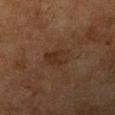Longest diameter approximately 3.5 mm. On the left upper arm. A roughly 15 mm field-of-view crop from a total-body skin photograph. A female patient aged around 60. This is a cross-polarized tile.